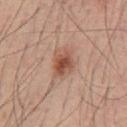The lesion was photographed on a routine skin check and not biopsied; there is no pathology result.
A 15 mm close-up tile from a total-body photography series done for melanoma screening.
The tile uses white-light illumination.
The lesion is located on the chest.
A male subject, approximately 45 years of age.
An algorithmic analysis of the crop reported a lesion area of about 8 mm² and a shape-asymmetry score of about 0.25 (0 = symmetric). And it measured radial color variation of about 1.5.